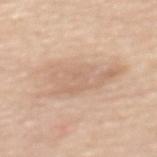Assessment: The lesion was tiled from a total-body skin photograph and was not biopsied. Context: Located on the mid back. Longest diameter approximately 8 mm. A female patient, aged 63–67. This is a white-light tile. A close-up tile cropped from a whole-body skin photograph, about 15 mm across.